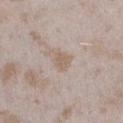Q: Is there a histopathology result?
A: catalogued during a skin exam; not biopsied